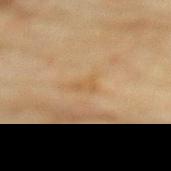biopsy_status: not biopsied; imaged during a skin examination
lighting: cross-polarized
patient:
  sex: female
  age_approx: 80
site: upper back
image:
  source: total-body photography crop
  field_of_view_mm: 15